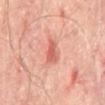Imaged during a routine full-body skin examination; the lesion was not biopsied and no histopathology is available.
A region of skin cropped from a whole-body photographic capture, roughly 15 mm wide.
The subject is a male aged 63–67.
This is a cross-polarized tile.
Automated tile analysis of the lesion measured a lesion color around L≈59 a*≈31 b*≈31 in CIELAB, about 11 CIELAB-L* units darker than the surrounding skin, and a normalized lesion–skin contrast near 7. The software also gave a border-irregularity rating of about 3/10, a within-lesion color-variation index near 2/10, and peripheral color asymmetry of about 0.5.
The lesion is on the mid back.